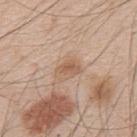follow-up: imaged on a skin check; not biopsied | patient: male, in their 50s | size: ≈3 mm | automated lesion analysis: an eccentricity of roughly 0.8 and a shape-asymmetry score of about 0.3 (0 = symmetric); a classifier nevus-likeness of about 0/100 and lesion-presence confidence of about 100/100 | tile lighting: white-light | body site: the upper back | acquisition: 15 mm crop, total-body photography.